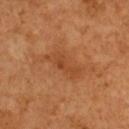Findings:
- biopsy status — total-body-photography surveillance lesion; no biopsy
- lesion diameter — about 5 mm
- image — ~15 mm tile from a whole-body skin photo
- location — the upper back
- subject — female, in their mid- to late 50s